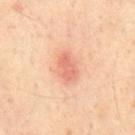Notes:
* follow-up: no biopsy performed (imaged during a skin exam)
* acquisition: ~15 mm tile from a whole-body skin photo
* anatomic site: the chest
* patient: male, approximately 65 years of age